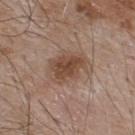Clinical impression: This lesion was catalogued during total-body skin photography and was not selected for biopsy.